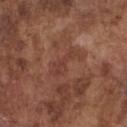Q: Was a biopsy performed?
A: catalogued during a skin exam; not biopsied
Q: How was this image acquired?
A: total-body-photography crop, ~15 mm field of view
Q: Who is the patient?
A: male, approximately 75 years of age
Q: Lesion size?
A: ~4.5 mm (longest diameter)
Q: Where on the body is the lesion?
A: the front of the torso